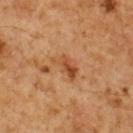Captured during whole-body skin photography for melanoma surveillance; the lesion was not biopsied. A close-up tile cropped from a whole-body skin photograph, about 15 mm across. The patient is a male about 60 years old. On the upper back.